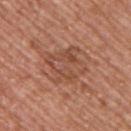This lesion was catalogued during total-body skin photography and was not selected for biopsy. Captured under white-light illumination. A roughly 15 mm field-of-view crop from a total-body skin photograph. A male subject, aged around 70. The lesion is on the upper back. Longest diameter approximately 5.5 mm. An algorithmic analysis of the crop reported a mean CIELAB color near L≈49 a*≈23 b*≈30 and a normalized lesion–skin contrast near 5.5. The software also gave a border-irregularity rating of about 4.5/10 and peripheral color asymmetry of about 2.